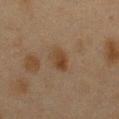Impression:
No biopsy was performed on this lesion — it was imaged during a full skin examination and was not determined to be concerning.
Acquisition and patient details:
A lesion tile, about 15 mm wide, cut from a 3D total-body photograph. On the chest. A female patient, aged approximately 40. Measured at roughly 3 mm in maximum diameter.